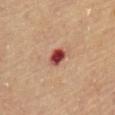Assessment: No biopsy was performed on this lesion — it was imaged during a full skin examination and was not determined to be concerning.